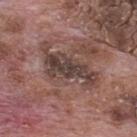No biopsy was performed on this lesion — it was imaged during a full skin examination and was not determined to be concerning. The lesion-visualizer software estimated an average lesion color of about L≈41 a*≈17 b*≈20 (CIELAB), about 10 CIELAB-L* units darker than the surrounding skin, and a normalized lesion–skin contrast near 8.5. A male patient aged approximately 70. The lesion is on the mid back. About 7.5 mm across. The tile uses white-light illumination. Cropped from a total-body skin-imaging series; the visible field is about 15 mm.